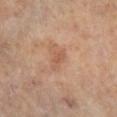notes: total-body-photography surveillance lesion; no biopsy | illumination: cross-polarized | acquisition: ~15 mm tile from a whole-body skin photo | lesion diameter: about 2.5 mm | body site: the leg | automated lesion analysis: a lesion area of about 3 mm² and an outline eccentricity of about 0.85 (0 = round, 1 = elongated); a mean CIELAB color near L≈55 a*≈22 b*≈32, roughly 7 lightness units darker than nearby skin, and a normalized lesion–skin contrast near 5; a border-irregularity rating of about 3.5/10, a color-variation rating of about 1/10, and peripheral color asymmetry of about 0.5 | patient: female, approximately 60 years of age.